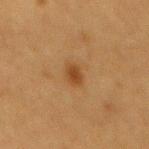Q: Was this lesion biopsied?
A: total-body-photography surveillance lesion; no biopsy
Q: Lesion location?
A: the back
Q: What did automated image analysis measure?
A: an average lesion color of about L≈37 a*≈18 b*≈34 (CIELAB), a lesion–skin lightness drop of about 8, and a lesion-to-skin contrast of about 7.5 (normalized; higher = more distinct)
Q: How large is the lesion?
A: ~2.5 mm (longest diameter)
Q: Illumination type?
A: cross-polarized
Q: Patient demographics?
A: female, in their mid-50s
Q: What is the imaging modality?
A: ~15 mm crop, total-body skin-cancer survey